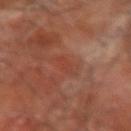This image is a 15 mm lesion crop taken from a total-body photograph.
The lesion-visualizer software estimated an area of roughly 3 mm², a shape eccentricity near 0.95, and a symmetry-axis asymmetry near 0.25. It also reported a mean CIELAB color near L≈41 a*≈26 b*≈30, roughly 5 lightness units darker than nearby skin, and a lesion-to-skin contrast of about 4.5 (normalized; higher = more distinct). And it measured border irregularity of about 3 on a 0–10 scale, a within-lesion color-variation index near 0/10, and peripheral color asymmetry of about 0. The software also gave an automated nevus-likeness rating near 0 out of 100.
Imaged with cross-polarized lighting.
Located on the right forearm.
A male subject, aged 63 to 67.